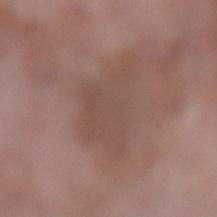Q: Who is the patient?
A: female, aged around 70
Q: How was this image acquired?
A: ~15 mm tile from a whole-body skin photo
Q: What did automated image analysis measure?
A: an eccentricity of roughly 0.8 and a shape-asymmetry score of about 0.4 (0 = symmetric); a border-irregularity index near 5/10, a color-variation rating of about 2/10, and peripheral color asymmetry of about 0.5; a nevus-likeness score of about 0/100 and a detector confidence of about 100 out of 100 that the crop contains a lesion
Q: What is the anatomic site?
A: the left lower leg
Q: How large is the lesion?
A: ~7 mm (longest diameter)